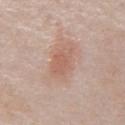Assessment: Captured during whole-body skin photography for melanoma surveillance; the lesion was not biopsied. Context: Located on the front of the torso. A male subject, aged around 75. A roughly 15 mm field-of-view crop from a total-body skin photograph.